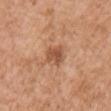The lesion was tiled from a total-body skin photograph and was not biopsied. From the chest. The patient is a male in their 80s. This is a white-light tile. Approximately 3 mm at its widest. A region of skin cropped from a whole-body photographic capture, roughly 15 mm wide.